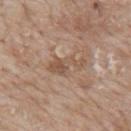Case summary:
– workup: total-body-photography surveillance lesion; no biopsy
– tile lighting: white-light
– anatomic site: the mid back
– patient: male, in their mid-60s
– imaging modality: ~15 mm tile from a whole-body skin photo